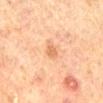{
  "biopsy_status": "not biopsied; imaged during a skin examination",
  "image": {
    "source": "total-body photography crop",
    "field_of_view_mm": 15
  },
  "patient": {
    "sex": "male",
    "age_approx": 70
  },
  "site": "mid back",
  "automated_metrics": {
    "area_mm2_approx": 3.0,
    "eccentricity": 0.8,
    "shape_asymmetry": 0.3,
    "cielab_L": 56,
    "cielab_a": 22,
    "cielab_b": 34,
    "vs_skin_darker_L": 8.0,
    "vs_skin_contrast_norm": 6.0,
    "nevus_likeness_0_100": 20
  },
  "lighting": "cross-polarized"
}